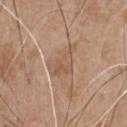<tbp_lesion>
  <biopsy_status>not biopsied; imaged during a skin examination</biopsy_status>
  <site>chest</site>
  <patient>
    <sex>male</sex>
    <age_approx>65</age_approx>
  </patient>
  <lighting>white-light</lighting>
  <lesion_size>
    <long_diameter_mm_approx>4.5</long_diameter_mm_approx>
  </lesion_size>
  <image>
    <source>total-body photography crop</source>
    <field_of_view_mm>15</field_of_view_mm>
  </image>
  <automated_metrics>
    <vs_skin_darker_L>7.0</vs_skin_darker_L>
    <vs_skin_contrast_norm>5.0</vs_skin_contrast_norm>
    <color_variation_0_10>3.5</color_variation_0_10>
    <peripheral_color_asymmetry>1.5</peripheral_color_asymmetry>
    <nevus_likeness_0_100>0</nevus_likeness_0_100>
  </automated_metrics>
</tbp_lesion>